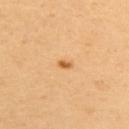notes: imaged on a skin check; not biopsied
acquisition: ~15 mm crop, total-body skin-cancer survey
location: the upper back
automated lesion analysis: a shape eccentricity near 0.8 and a symmetry-axis asymmetry near 0.2; a border-irregularity rating of about 1.5/10, a color-variation rating of about 0/10, and a peripheral color-asymmetry measure near 0; a nevus-likeness score of about 95/100 and lesion-presence confidence of about 100/100
patient: female, roughly 40 years of age
lighting: cross-polarized